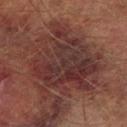Q: What did automated image analysis measure?
A: a lesion area of about 50 mm², a shape eccentricity near 0.25, and a symmetry-axis asymmetry near 0.3; a lesion-to-skin contrast of about 9 (normalized; higher = more distinct); a classifier nevus-likeness of about 0/100
Q: What are the patient's age and sex?
A: male, in their mid-70s
Q: Illumination type?
A: cross-polarized illumination
Q: Where on the body is the lesion?
A: the left lower leg
Q: What kind of image is this?
A: ~15 mm crop, total-body skin-cancer survey